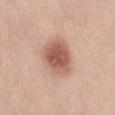Q: Was this lesion biopsied?
A: total-body-photography surveillance lesion; no biopsy
Q: What is the lesion's diameter?
A: ≈4.5 mm
Q: How was this image acquired?
A: 15 mm crop, total-body photography
Q: Patient demographics?
A: female, aged approximately 20
Q: Where on the body is the lesion?
A: the right thigh
Q: Automated lesion metrics?
A: a lesion area of about 15 mm², an outline eccentricity of about 0.4 (0 = round, 1 = elongated), and two-axis asymmetry of about 0.2; a lesion color around L≈58 a*≈23 b*≈28 in CIELAB and about 13 CIELAB-L* units darker than the surrounding skin; a border-irregularity index near 2/10 and a color-variation rating of about 4/10